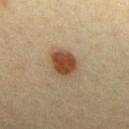<tbp_lesion>
  <biopsy_status>not biopsied; imaged during a skin examination</biopsy_status>
  <lesion_size>
    <long_diameter_mm_approx>3.5</long_diameter_mm_approx>
  </lesion_size>
  <automated_metrics>
    <border_irregularity_0_10>1.5</border_irregularity_0_10>
    <color_variation_0_10>4.0</color_variation_0_10>
    <peripheral_color_asymmetry>1.5</peripheral_color_asymmetry>
  </automated_metrics>
  <site>upper back</site>
  <patient>
    <sex>male</sex>
    <age_approx>40</age_approx>
  </patient>
  <image>
    <source>total-body photography crop</source>
    <field_of_view_mm>15</field_of_view_mm>
  </image>
  <lighting>cross-polarized</lighting>
</tbp_lesion>